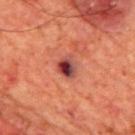workup = catalogued during a skin exam; not biopsied
image source = ~15 mm crop, total-body skin-cancer survey
anatomic site = the mid back
patient = male, roughly 65 years of age
lesion size = ~3 mm (longest diameter)
tile lighting = cross-polarized illumination